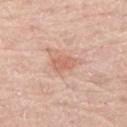{"biopsy_status": "not biopsied; imaged during a skin examination"}